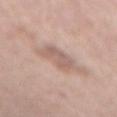  biopsy_status: not biopsied; imaged during a skin examination